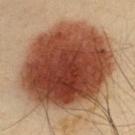  biopsy_status: not biopsied; imaged during a skin examination
  lighting: cross-polarized
  patient:
    sex: male
    age_approx: 35
  site: back
  image:
    source: total-body photography crop
    field_of_view_mm: 15